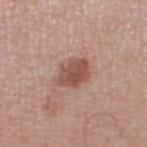{
  "biopsy_status": "not biopsied; imaged during a skin examination",
  "lighting": "white-light",
  "automated_metrics": {
    "cielab_L": 51,
    "cielab_a": 22,
    "cielab_b": 25,
    "vs_skin_darker_L": 12.0,
    "vs_skin_contrast_norm": 8.5,
    "color_variation_0_10": 3.0,
    "peripheral_color_asymmetry": 1.0,
    "nevus_likeness_0_100": 45,
    "lesion_detection_confidence_0_100": 100
  },
  "lesion_size": {
    "long_diameter_mm_approx": 4.0
  },
  "patient": {
    "sex": "male",
    "age_approx": 70
  },
  "image": {
    "source": "total-body photography crop",
    "field_of_view_mm": 15
  },
  "site": "leg"
}